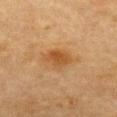Q: Is there a histopathology result?
A: no biopsy performed (imaged during a skin exam)
Q: What are the patient's age and sex?
A: male, aged 83 or over
Q: What is the lesion's diameter?
A: about 3.5 mm
Q: What is the anatomic site?
A: the front of the torso
Q: How was the tile lit?
A: cross-polarized illumination
Q: What is the imaging modality?
A: 15 mm crop, total-body photography